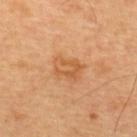- biopsy status: total-body-photography surveillance lesion; no biopsy
- lesion diameter: ~3.5 mm (longest diameter)
- subject: male, about 60 years old
- tile lighting: cross-polarized illumination
- acquisition: total-body-photography crop, ~15 mm field of view
- body site: the upper back